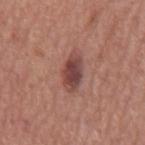{"biopsy_status": "not biopsied; imaged during a skin examination", "lesion_size": {"long_diameter_mm_approx": 4.0}, "automated_metrics": {"cielab_L": 44, "cielab_a": 23, "cielab_b": 23, "vs_skin_contrast_norm": 9.5, "border_irregularity_0_10": 2.0, "color_variation_0_10": 4.0}, "patient": {"sex": "male", "age_approx": 75}, "site": "mid back", "lighting": "white-light", "image": {"source": "total-body photography crop", "field_of_view_mm": 15}}